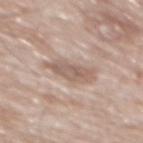workup = imaged on a skin check; not biopsied | acquisition = total-body-photography crop, ~15 mm field of view | patient = male, aged 78–82 | automated lesion analysis = a lesion color around L≈59 a*≈16 b*≈24 in CIELAB, roughly 10 lightness units darker than nearby skin, and a normalized border contrast of about 6.5; a border-irregularity index near 3/10, a within-lesion color-variation index near 4/10, and peripheral color asymmetry of about 1.5; a classifier nevus-likeness of about 0/100 and lesion-presence confidence of about 85/100 | illumination = white-light illumination | anatomic site = the mid back | size = about 4.5 mm.